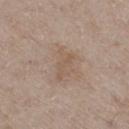workup — catalogued during a skin exam; not biopsied | image-analysis metrics — about 6 CIELAB-L* units darker than the surrounding skin; a border-irregularity index near 4.5/10 and radial color variation of about 0.5; a classifier nevus-likeness of about 0/100 and a detector confidence of about 100 out of 100 that the crop contains a lesion | image source — ~15 mm crop, total-body skin-cancer survey | anatomic site — the right thigh | lesion diameter — ≈4 mm | lighting — white-light illumination | patient — male, aged around 50.